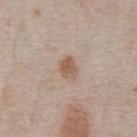Q: Was this lesion biopsied?
A: imaged on a skin check; not biopsied
Q: What did automated image analysis measure?
A: an average lesion color of about L≈57 a*≈18 b*≈28 (CIELAB), about 10 CIELAB-L* units darker than the surrounding skin, and a normalized border contrast of about 7.5; border irregularity of about 2 on a 0–10 scale, internal color variation of about 3 on a 0–10 scale, and a peripheral color-asymmetry measure near 1
Q: Lesion location?
A: the chest
Q: What is the lesion's diameter?
A: ≈2.5 mm
Q: Illumination type?
A: white-light illumination
Q: What kind of image is this?
A: ~15 mm crop, total-body skin-cancer survey
Q: Who is the patient?
A: male, about 75 years old